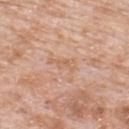Captured during whole-body skin photography for melanoma surveillance; the lesion was not biopsied.
The lesion's longest dimension is about 3.5 mm.
The lesion-visualizer software estimated a border-irregularity index near 6/10, a color-variation rating of about 0/10, and radial color variation of about 0. The software also gave a nevus-likeness score of about 0/100 and a detector confidence of about 100 out of 100 that the crop contains a lesion.
A 15 mm close-up extracted from a 3D total-body photography capture.
Imaged with white-light lighting.
A male patient, about 80 years old.
The lesion is located on the upper back.